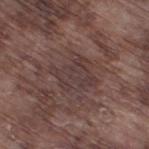acquisition: ~15 mm crop, total-body skin-cancer survey
illumination: white-light illumination
body site: the right thigh
subject: male, roughly 75 years of age
TBP lesion metrics: an area of roughly 12 mm², an outline eccentricity of about 0.5 (0 = round, 1 = elongated), and two-axis asymmetry of about 0.35; a mean CIELAB color near L≈37 a*≈16 b*≈17, roughly 6 lightness units darker than nearby skin, and a lesion-to-skin contrast of about 5.5 (normalized; higher = more distinct); an automated nevus-likeness rating near 0 out of 100 and lesion-presence confidence of about 50/100